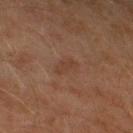This lesion was catalogued during total-body skin photography and was not selected for biopsy.
The patient is a male aged 58 to 62.
About 2.5 mm across.
The total-body-photography lesion software estimated a nevus-likeness score of about 15/100 and lesion-presence confidence of about 100/100.
The lesion is located on the right thigh.
Imaged with cross-polarized lighting.
Cropped from a whole-body photographic skin survey; the tile spans about 15 mm.